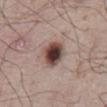A roughly 15 mm field-of-view crop from a total-body skin photograph. On the abdomen. Longest diameter approximately 3.5 mm. A male patient, in their mid-60s.